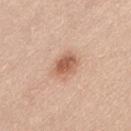Notes:
- biopsy status: catalogued during a skin exam; not biopsied